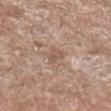Findings:
– biopsy status · total-body-photography surveillance lesion; no biopsy
– location · the left forearm
– acquisition · ~15 mm tile from a whole-body skin photo
– automated lesion analysis · a lesion color around L≈55 a*≈17 b*≈26 in CIELAB and a lesion–skin lightness drop of about 8; border irregularity of about 2.5 on a 0–10 scale, internal color variation of about 2.5 on a 0–10 scale, and a peripheral color-asymmetry measure near 1; a lesion-detection confidence of about 100/100
– subject · male, aged 48 to 52